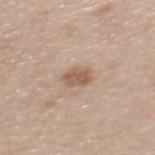Clinical impression:
This lesion was catalogued during total-body skin photography and was not selected for biopsy.
Context:
The total-body-photography lesion software estimated a lesion color around L≈58 a*≈17 b*≈30 in CIELAB, a lesion–skin lightness drop of about 10, and a normalized border contrast of about 7.5. The software also gave a border-irregularity index near 2/10, a within-lesion color-variation index near 2/10, and peripheral color asymmetry of about 0.5. The lesion is located on the back. The lesion's longest dimension is about 3.5 mm. A 15 mm close-up tile from a total-body photography series done for melanoma screening. Imaged with white-light lighting. The patient is a female aged 43 to 47.